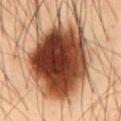Assessment:
The lesion was photographed on a routine skin check and not biopsied; there is no pathology result.
Background:
The tile uses cross-polarized illumination. Located on the abdomen. The lesion's longest dimension is about 9.5 mm. The lesion-visualizer software estimated an average lesion color of about L≈36 a*≈20 b*≈28 (CIELAB) and a lesion-to-skin contrast of about 16 (normalized; higher = more distinct). The analysis additionally found an automated nevus-likeness rating near 95 out of 100. A male subject aged 53 to 57. A close-up tile cropped from a whole-body skin photograph, about 15 mm across.